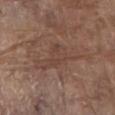Captured during whole-body skin photography for melanoma surveillance; the lesion was not biopsied. A close-up tile cropped from a whole-body skin photograph, about 15 mm across. Automated image analysis of the tile measured an eccentricity of roughly 0.85 and two-axis asymmetry of about 0.6. A male subject, roughly 80 years of age. The lesion is on the abdomen. Approximately 6.5 mm at its widest.